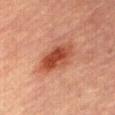Part of a total-body skin-imaging series; this lesion was reviewed on a skin check and was not flagged for biopsy. The recorded lesion diameter is about 5 mm. Automated tile analysis of the lesion measured a lesion area of about 12 mm², a shape eccentricity near 0.85, and a shape-asymmetry score of about 0.2 (0 = symmetric). The analysis additionally found a lesion–skin lightness drop of about 12. And it measured border irregularity of about 2.5 on a 0–10 scale, internal color variation of about 6 on a 0–10 scale, and radial color variation of about 2. The analysis additionally found an automated nevus-likeness rating near 95 out of 100 and a lesion-detection confidence of about 100/100. A female subject, in their 60s. This is a cross-polarized tile. A 15 mm close-up tile from a total-body photography series done for melanoma screening. The lesion is located on the front of the torso.